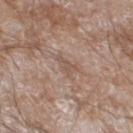follow-up: no biopsy performed (imaged during a skin exam); anatomic site: the left lower leg; acquisition: ~15 mm tile from a whole-body skin photo; patient: male, aged 58 to 62.